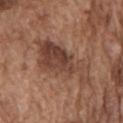No biopsy was performed on this lesion — it was imaged during a full skin examination and was not determined to be concerning. The lesion is located on the front of the torso. A close-up tile cropped from a whole-body skin photograph, about 15 mm across. The tile uses white-light illumination. A male patient, aged around 75. Approximately 7.5 mm at its widest.